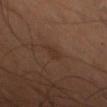Assessment: The lesion was photographed on a routine skin check and not biopsied; there is no pathology result. Acquisition and patient details: Located on the leg. Cropped from a whole-body photographic skin survey; the tile spans about 15 mm. A male patient roughly 50 years of age.